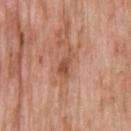  biopsy_status: not biopsied; imaged during a skin examination
  image:
    source: total-body photography crop
    field_of_view_mm: 15
  automated_metrics:
    eccentricity: 0.9
    shape_asymmetry: 0.4
    cielab_L: 52
    cielab_a: 24
    cielab_b: 33
    vs_skin_contrast_norm: 7.0
    border_irregularity_0_10: 4.5
    color_variation_0_10: 2.0
    peripheral_color_asymmetry: 0.5
    nevus_likeness_0_100: 5
    lesion_detection_confidence_0_100: 100
  lighting: white-light
  patient:
    sex: male
    age_approx: 60
  lesion_size:
    long_diameter_mm_approx: 3.5
  site: upper back